On the arm · this image is a 15 mm lesion crop taken from a total-body photograph · the subject is a female roughly 60 years of age:
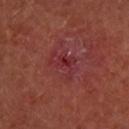Automated image analysis of the tile measured a lesion color around L≈31 a*≈31 b*≈21 in CIELAB, a lesion–skin lightness drop of about 5, and a lesion-to-skin contrast of about 5.5 (normalized; higher = more distinct). And it measured a border-irregularity index near 7.5/10 and radial color variation of about 1. It also reported a classifier nevus-likeness of about 0/100 and lesion-presence confidence of about 75/100. Measured at roughly 4 mm in maximum diameter. Captured under cross-polarized illumination. The lesion was biopsied, and histopathology showed an actinic keratosis (borderline).